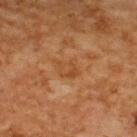{"biopsy_status": "not biopsied; imaged during a skin examination", "lesion_size": {"long_diameter_mm_approx": 2.5}, "site": "upper back", "automated_metrics": {"area_mm2_approx": 5.0, "eccentricity": 0.65, "shape_asymmetry": 0.3, "cielab_L": 44, "cielab_a": 21, "cielab_b": 36, "vs_skin_darker_L": 6.0, "vs_skin_contrast_norm": 5.0, "color_variation_0_10": 3.5, "peripheral_color_asymmetry": 1.0, "nevus_likeness_0_100": 0, "lesion_detection_confidence_0_100": 100}, "image": {"source": "total-body photography crop", "field_of_view_mm": 15}, "patient": {"sex": "male", "age_approx": 60}}